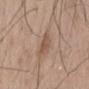| field | value |
|---|---|
| biopsy status | catalogued during a skin exam; not biopsied |
| subject | male, about 65 years old |
| lesion size | about 2.5 mm |
| imaging modality | total-body-photography crop, ~15 mm field of view |
| body site | the mid back |